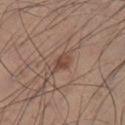No biopsy was performed on this lesion — it was imaged during a full skin examination and was not determined to be concerning.
On the left lower leg.
Approximately 2.5 mm at its widest.
A male patient, aged 33 to 37.
Automated tile analysis of the lesion measured internal color variation of about 3 on a 0–10 scale and peripheral color asymmetry of about 1. It also reported an automated nevus-likeness rating near 80 out of 100 and a lesion-detection confidence of about 100/100.
Captured under white-light illumination.
A 15 mm close-up tile from a total-body photography series done for melanoma screening.